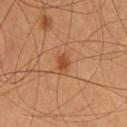biopsy_status: not biopsied; imaged during a skin examination
lesion_size:
  long_diameter_mm_approx: 2.5
patient:
  sex: female
  age_approx: 30
automated_metrics:
  cielab_L: 46
  cielab_a: 24
  cielab_b: 36
  vs_skin_darker_L: 9.0
  vs_skin_contrast_norm: 7.0
  border_irregularity_0_10: 2.5
  color_variation_0_10: 2.0
  peripheral_color_asymmetry: 0.5
image:
  source: total-body photography crop
  field_of_view_mm: 15
site: upper back
lighting: cross-polarized